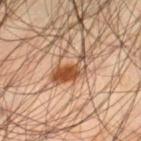Imaged during a routine full-body skin examination; the lesion was not biopsied and no histopathology is available.
The patient is a male aged 43 to 47.
From the left thigh.
This image is a 15 mm lesion crop taken from a total-body photograph.